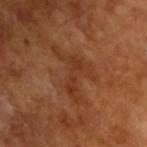Impression:
The lesion was photographed on a routine skin check and not biopsied; there is no pathology result.
Background:
A male subject approximately 65 years of age. Approximately 6.5 mm at its widest. Automated image analysis of the tile measured a lesion color around L≈35 a*≈24 b*≈32 in CIELAB and a normalized border contrast of about 5.5. It also reported a nevus-likeness score of about 0/100 and lesion-presence confidence of about 90/100. The tile uses cross-polarized illumination. A lesion tile, about 15 mm wide, cut from a 3D total-body photograph.